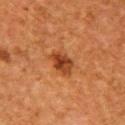Impression:
Captured during whole-body skin photography for melanoma surveillance; the lesion was not biopsied.
Clinical summary:
A female patient, aged around 50. Longest diameter approximately 3.5 mm. Captured under cross-polarized illumination. Automated image analysis of the tile measured a classifier nevus-likeness of about 90/100 and a detector confidence of about 100 out of 100 that the crop contains a lesion. On the right upper arm. A 15 mm crop from a total-body photograph taken for skin-cancer surveillance.